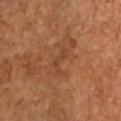follow-up: no biopsy performed (imaged during a skin exam)